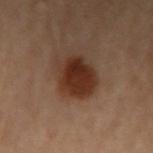Q: Was this lesion biopsied?
A: catalogued during a skin exam; not biopsied
Q: Illumination type?
A: cross-polarized illumination
Q: What did automated image analysis measure?
A: an automated nevus-likeness rating near 100 out of 100 and lesion-presence confidence of about 100/100
Q: What is the anatomic site?
A: the arm
Q: How large is the lesion?
A: ≈5 mm
Q: Who is the patient?
A: female, about 60 years old
Q: What kind of image is this?
A: ~15 mm crop, total-body skin-cancer survey